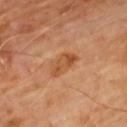Context:
A male patient in their 60s. A roughly 15 mm field-of-view crop from a total-body skin photograph. The tile uses cross-polarized illumination. Located on the back. Approximately 4 mm at its widest. An algorithmic analysis of the crop reported a footprint of about 5.5 mm² and an outline eccentricity of about 0.9 (0 = round, 1 = elongated). The analysis additionally found a mean CIELAB color near L≈49 a*≈24 b*≈38, a lesion–skin lightness drop of about 9, and a normalized lesion–skin contrast near 7.